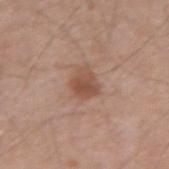Clinical impression: Recorded during total-body skin imaging; not selected for excision or biopsy. Image and clinical context: A male subject, aged approximately 50. The lesion is located on the right upper arm. A 15 mm close-up extracted from a 3D total-body photography capture.